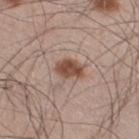• notes — total-body-photography surveillance lesion; no biopsy
• subject — male, about 45 years old
• diameter — ~3 mm (longest diameter)
• lighting — white-light
• body site — the right thigh
• image — 15 mm crop, total-body photography
• image-analysis metrics — a footprint of about 5.5 mm², an outline eccentricity of about 0.7 (0 = round, 1 = elongated), and a symmetry-axis asymmetry near 0.15; a lesion–skin lightness drop of about 14 and a normalized lesion–skin contrast near 10; a classifier nevus-likeness of about 95/100